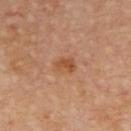location: the back | acquisition: 15 mm crop, total-body photography | subject: female, aged 63–67 | lighting: cross-polarized.